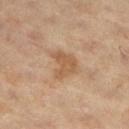The lesion was photographed on a routine skin check and not biopsied; there is no pathology result.
A region of skin cropped from a whole-body photographic capture, roughly 15 mm wide.
A male subject, aged around 60.
Imaged with cross-polarized lighting.
About 3.5 mm across.
From the right thigh.